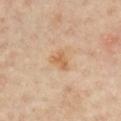{
  "biopsy_status": "not biopsied; imaged during a skin examination",
  "lesion_size": {
    "long_diameter_mm_approx": 2.5
  },
  "automated_metrics": {
    "area_mm2_approx": 4.5,
    "cielab_L": 62,
    "cielab_a": 20,
    "cielab_b": 37,
    "vs_skin_darker_L": 8.0,
    "vs_skin_contrast_norm": 6.5,
    "nevus_likeness_0_100": 5
  },
  "lighting": "cross-polarized",
  "image": {
    "source": "total-body photography crop",
    "field_of_view_mm": 15
  },
  "patient": {
    "sex": "male",
    "age_approx": 65
  },
  "site": "chest"
}